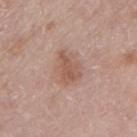A lesion tile, about 15 mm wide, cut from a 3D total-body photograph. Longest diameter approximately 3.5 mm. A male subject, aged 78 to 82. Automated image analysis of the tile measured lesion-presence confidence of about 100/100. On the left upper arm. This is a white-light tile.